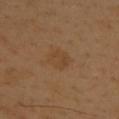biopsy_status: not biopsied; imaged during a skin examination
image:
  source: total-body photography crop
  field_of_view_mm: 15
lighting: cross-polarized
site: upper back
lesion_size:
  long_diameter_mm_approx: 3.0
automated_metrics:
  area_mm2_approx: 6.0
  shape_asymmetry: 0.2
  cielab_L: 42
  cielab_a: 17
  cielab_b: 33
  vs_skin_darker_L: 5.0
  vs_skin_contrast_norm: 5.0
  nevus_likeness_0_100: 10
  lesion_detection_confidence_0_100: 100
patient:
  sex: male
  age_approx: 40